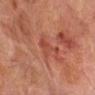notes=imaged on a skin check; not biopsied
image-analysis metrics=internal color variation of about 2.5 on a 0–10 scale and a peripheral color-asymmetry measure near 0.5
image=~15 mm crop, total-body skin-cancer survey
subject=male, about 70 years old
lighting=cross-polarized illumination
lesion diameter=≈4 mm
location=the right forearm